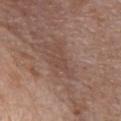<record>
  <site>front of the torso</site>
  <image>
    <source>total-body photography crop</source>
    <field_of_view_mm>15</field_of_view_mm>
  </image>
  <patient>
    <sex>male</sex>
    <age_approx>80</age_approx>
  </patient>
  <lighting>white-light</lighting>
  <lesion_size>
    <long_diameter_mm_approx>5.0</long_diameter_mm_approx>
  </lesion_size>
</record>